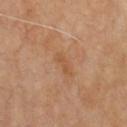<record>
<image>
  <source>total-body photography crop</source>
  <field_of_view_mm>15</field_of_view_mm>
</image>
<lesion_size>
  <long_diameter_mm_approx>3.0</long_diameter_mm_approx>
</lesion_size>
<patient>
  <sex>male</sex>
  <age_approx>70</age_approx>
</patient>
<lighting>cross-polarized</lighting>
<site>chest</site>
<automated_metrics>
  <area_mm2_approx>2.5</area_mm2_approx>
  <eccentricity>0.95</eccentricity>
  <shape_asymmetry>0.45</shape_asymmetry>
  <cielab_L>51</cielab_L>
  <cielab_a>21</cielab_a>
  <cielab_b>35</cielab_b>
  <vs_skin_darker_L>6.0</vs_skin_darker_L>
  <vs_skin_contrast_norm>5.0</vs_skin_contrast_norm>
</automated_metrics>
</record>